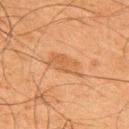  biopsy_status: not biopsied; imaged during a skin examination
  image:
    source: total-body photography crop
    field_of_view_mm: 15
  patient:
    sex: male
    age_approx: 65
  lighting: cross-polarized
  site: upper back